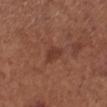Recorded during total-body skin imaging; not selected for excision or biopsy. The patient is a female in their mid-50s. Automated image analysis of the tile measured a lesion area of about 3.5 mm², a shape eccentricity near 0.75, and a symmetry-axis asymmetry near 0.25. The software also gave an average lesion color of about L≈37 a*≈22 b*≈27 (CIELAB) and about 7 CIELAB-L* units darker than the surrounding skin. This image is a 15 mm lesion crop taken from a total-body photograph. The lesion is on the leg.